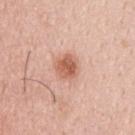Imaged during a routine full-body skin examination; the lesion was not biopsied and no histopathology is available. A lesion tile, about 15 mm wide, cut from a 3D total-body photograph. Automated tile analysis of the lesion measured a mean CIELAB color near L≈60 a*≈25 b*≈32 and a lesion–skin lightness drop of about 12. A male patient, aged approximately 35. Located on the upper back. Captured under white-light illumination.